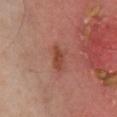Q: Patient demographics?
A: male, approximately 65 years of age
Q: How was the tile lit?
A: cross-polarized
Q: What is the anatomic site?
A: the chest
Q: Lesion size?
A: about 3 mm
Q: Automated lesion metrics?
A: a border-irregularity rating of about 3/10, a color-variation rating of about 2/10, and peripheral color asymmetry of about 0.5; a nevus-likeness score of about 50/100 and a lesion-detection confidence of about 100/100
Q: What kind of image is this?
A: ~15 mm tile from a whole-body skin photo